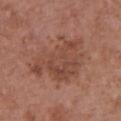Assessment:
Captured during whole-body skin photography for melanoma surveillance; the lesion was not biopsied.
Image and clinical context:
The subject is a female in their mid- to late 70s. Located on the chest. Cropped from a whole-body photographic skin survey; the tile spans about 15 mm.